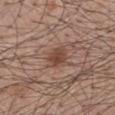<tbp_lesion>
  <biopsy_status>not biopsied; imaged during a skin examination</biopsy_status>
  <image>
    <source>total-body photography crop</source>
    <field_of_view_mm>15</field_of_view_mm>
  </image>
  <automated_metrics>
    <area_mm2_approx>6.0</area_mm2_approx>
    <eccentricity>0.7</eccentricity>
    <shape_asymmetry>0.2</shape_asymmetry>
    <cielab_L>45</cielab_L>
    <cielab_a>20</cielab_a>
    <cielab_b>26</cielab_b>
    <vs_skin_darker_L>9.0</vs_skin_darker_L>
    <vs_skin_contrast_norm>7.5</vs_skin_contrast_norm>
    <border_irregularity_0_10>2.0</border_irregularity_0_10>
    <color_variation_0_10>3.0</color_variation_0_10>
    <peripheral_color_asymmetry>1.0</peripheral_color_asymmetry>
    <nevus_likeness_0_100>90</nevus_likeness_0_100>
    <lesion_detection_confidence_0_100>100</lesion_detection_confidence_0_100>
  </automated_metrics>
  <patient>
    <sex>male</sex>
    <age_approx>50</age_approx>
  </patient>
  <site>mid back</site>
</tbp_lesion>